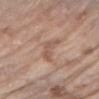workup: catalogued during a skin exam; not biopsied | tile lighting: white-light | body site: the right forearm | acquisition: 15 mm crop, total-body photography | subject: female, aged 68–72 | automated lesion analysis: a mean CIELAB color near L≈55 a*≈19 b*≈28, roughly 8 lightness units darker than nearby skin, and a normalized lesion–skin contrast near 5.5; a within-lesion color-variation index near 1.5/10 and a peripheral color-asymmetry measure near 0.5.